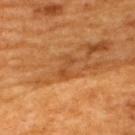Case summary:
* workup · total-body-photography surveillance lesion; no biopsy